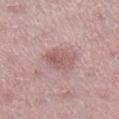The lesion was tiled from a total-body skin photograph and was not biopsied. An algorithmic analysis of the crop reported an average lesion color of about L≈57 a*≈22 b*≈20 (CIELAB), a lesion–skin lightness drop of about 9, and a lesion-to-skin contrast of about 6 (normalized; higher = more distinct). Captured under white-light illumination. On the right lower leg. Longest diameter approximately 4 mm. A close-up tile cropped from a whole-body skin photograph, about 15 mm across. A female subject aged around 45.